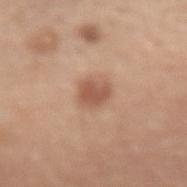Part of a total-body skin-imaging series; this lesion was reviewed on a skin check and was not flagged for biopsy.
A male patient aged 68–72.
Automated tile analysis of the lesion measured a symmetry-axis asymmetry near 0.25. The analysis additionally found a classifier nevus-likeness of about 85/100 and lesion-presence confidence of about 100/100.
From the lower back.
A roughly 15 mm field-of-view crop from a total-body skin photograph.
The lesion's longest dimension is about 2.5 mm.